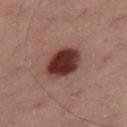- follow-up — imaged on a skin check; not biopsied
- acquisition — total-body-photography crop, ~15 mm field of view
- size — about 4.5 mm
- site — the right thigh
- automated metrics — an average lesion color of about L≈29 a*≈20 b*≈20 (CIELAB) and about 16 CIELAB-L* units darker than the surrounding skin; a nevus-likeness score of about 100/100
- subject — male, in their 50s
- tile lighting — cross-polarized illumination